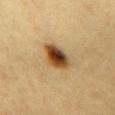The lesion was tiled from a total-body skin photograph and was not biopsied.
Located on the chest.
The lesion's longest dimension is about 4.5 mm.
A 15 mm close-up tile from a total-body photography series done for melanoma screening.
The patient is a female roughly 30 years of age.
The tile uses cross-polarized illumination.